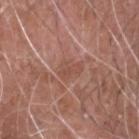biopsy status = no biopsy performed (imaged during a skin exam)
location = the head or neck
TBP lesion metrics = a normalized lesion–skin contrast near 5; a border-irregularity index near 3/10, internal color variation of about 2 on a 0–10 scale, and a peripheral color-asymmetry measure near 0.5; an automated nevus-likeness rating near 0 out of 100 and a lesion-detection confidence of about 70/100
diameter = ~3.5 mm (longest diameter)
imaging modality = total-body-photography crop, ~15 mm field of view
subject = male, in their mid- to late 70s
illumination = white-light